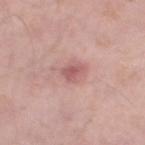This is a white-light tile.
The subject is a male aged 53 to 57.
From the left thigh.
The lesion-visualizer software estimated about 10 CIELAB-L* units darker than the surrounding skin. The analysis additionally found a border-irregularity rating of about 2.5/10, a color-variation rating of about 2/10, and peripheral color asymmetry of about 0.5. The analysis additionally found a nevus-likeness score of about 20/100 and a detector confidence of about 100 out of 100 that the crop contains a lesion.
Cropped from a whole-body photographic skin survey; the tile spans about 15 mm.
The recorded lesion diameter is about 3 mm.